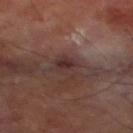Part of a total-body skin-imaging series; this lesion was reviewed on a skin check and was not flagged for biopsy.
This image is a 15 mm lesion crop taken from a total-body photograph.
On the leg.
A male patient about 70 years old.
The recorded lesion diameter is about 3.5 mm.
The lesion-visualizer software estimated a footprint of about 5.5 mm², a shape eccentricity near 0.65, and two-axis asymmetry of about 0.25. The analysis additionally found a mean CIELAB color near L≈31 a*≈18 b*≈18 and a lesion-to-skin contrast of about 7 (normalized; higher = more distinct).
The tile uses cross-polarized illumination.